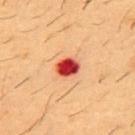The lesion was tiled from a total-body skin photograph and was not biopsied. An algorithmic analysis of the crop reported a lesion color around L≈42 a*≈40 b*≈33 in CIELAB, about 21 CIELAB-L* units darker than the surrounding skin, and a normalized border contrast of about 14.5. The patient is a male aged 53 to 57. A roughly 15 mm field-of-view crop from a total-body skin photograph. Located on the chest.